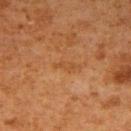workup: imaged on a skin check; not biopsied | imaging modality: 15 mm crop, total-body photography | lighting: cross-polarized illumination | patient: male, aged 58 to 62 | TBP lesion metrics: a lesion color around L≈41 a*≈20 b*≈35 in CIELAB, a lesion–skin lightness drop of about 5, and a normalized border contrast of about 5; a border-irregularity rating of about 4.5/10 and radial color variation of about 0 | anatomic site: the right upper arm | diameter: ~2.5 mm (longest diameter).